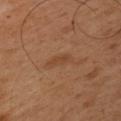Impression:
This lesion was catalogued during total-body skin photography and was not selected for biopsy.
Clinical summary:
From the left upper arm. Imaged with cross-polarized lighting. This image is a 15 mm lesion crop taken from a total-body photograph. The patient is a male aged 48 to 52.